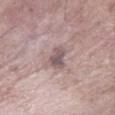Background: The lesion-visualizer software estimated an area of roughly 5 mm², an eccentricity of roughly 0.7, and two-axis asymmetry of about 0.3. It also reported border irregularity of about 3.5 on a 0–10 scale, a color-variation rating of about 3.5/10, and peripheral color asymmetry of about 1. The analysis additionally found a lesion-detection confidence of about 90/100. Located on the arm. A region of skin cropped from a whole-body photographic capture, roughly 15 mm wide. A male subject, aged approximately 60. The lesion's longest dimension is about 3 mm. Captured under white-light illumination.